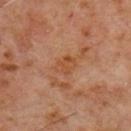Q: Is there a histopathology result?
A: total-body-photography surveillance lesion; no biopsy
Q: How large is the lesion?
A: about 2.5 mm
Q: What is the imaging modality?
A: ~15 mm tile from a whole-body skin photo
Q: What lighting was used for the tile?
A: cross-polarized illumination
Q: Who is the patient?
A: male, in their 60s
Q: Automated lesion metrics?
A: a mean CIELAB color near L≈45 a*≈23 b*≈34, about 6 CIELAB-L* units darker than the surrounding skin, and a normalized border contrast of about 6; a nevus-likeness score of about 0/100
Q: What is the anatomic site?
A: the front of the torso